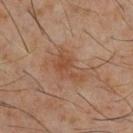An algorithmic analysis of the crop reported a lesion color around L≈44 a*≈20 b*≈30 in CIELAB and about 7 CIELAB-L* units darker than the surrounding skin. The software also gave a border-irregularity index near 5.5/10, a within-lesion color-variation index near 3/10, and peripheral color asymmetry of about 1. It also reported an automated nevus-likeness rating near 0 out of 100 and a lesion-detection confidence of about 100/100. A male patient, about 60 years old. The lesion is on the front of the torso. Captured under cross-polarized illumination. A 15 mm crop from a total-body photograph taken for skin-cancer surveillance. The recorded lesion diameter is about 4.5 mm.